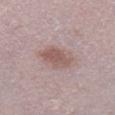Impression:
The lesion was tiled from a total-body skin photograph and was not biopsied.
Clinical summary:
The patient is a male about 50 years old. A lesion tile, about 15 mm wide, cut from a 3D total-body photograph. The tile uses white-light illumination. Measured at roughly 4 mm in maximum diameter. The lesion is located on the left lower leg.